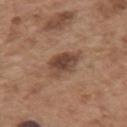A male subject, about 55 years old. A 15 mm crop from a total-body photograph taken for skin-cancer surveillance. About 4 mm across. The lesion is on the left upper arm.